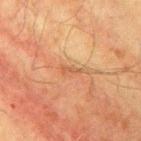biopsy_status: not biopsied; imaged during a skin examination
lesion_size:
  long_diameter_mm_approx: 2.5
image:
  source: total-body photography crop
  field_of_view_mm: 15
site: arm
patient:
  sex: male
  age_approx: 70